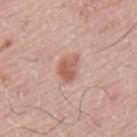Q: Was a biopsy performed?
A: total-body-photography surveillance lesion; no biopsy
Q: What is the imaging modality?
A: 15 mm crop, total-body photography
Q: Where on the body is the lesion?
A: the mid back
Q: What are the patient's age and sex?
A: male, aged approximately 75